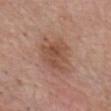{"lesion_size": {"long_diameter_mm_approx": 5.0}, "site": "front of the torso", "lighting": "white-light", "image": {"source": "total-body photography crop", "field_of_view_mm": 15}, "patient": {"sex": "male", "age_approx": 80}}